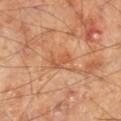Impression:
This lesion was catalogued during total-body skin photography and was not selected for biopsy.
Image and clinical context:
Automated image analysis of the tile measured a lesion area of about 4.5 mm², an outline eccentricity of about 0.8 (0 = round, 1 = elongated), and two-axis asymmetry of about 0.45. The analysis additionally found a detector confidence of about 100 out of 100 that the crop contains a lesion. Measured at roughly 3 mm in maximum diameter. A male subject, approximately 70 years of age. Located on the right lower leg. A roughly 15 mm field-of-view crop from a total-body skin photograph. Imaged with cross-polarized lighting.